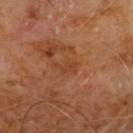notes: no biopsy performed (imaged during a skin exam) | body site: the chest | patient: male, about 60 years old | image source: ~15 mm crop, total-body skin-cancer survey | tile lighting: cross-polarized.